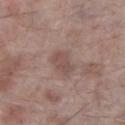| field | value |
|---|---|
| follow-up | total-body-photography surveillance lesion; no biopsy |
| lesion size | about 3 mm |
| automated metrics | a footprint of about 5.5 mm² and an outline eccentricity of about 0.8 (0 = round, 1 = elongated); a lesion–skin lightness drop of about 8 and a normalized border contrast of about 5.5; a nevus-likeness score of about 30/100 and a detector confidence of about 100 out of 100 that the crop contains a lesion |
| patient | male, in their 70s |
| lighting | white-light |
| acquisition | ~15 mm tile from a whole-body skin photo |
| site | the right lower leg |